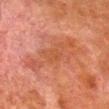workup: no biopsy performed (imaged during a skin exam) | body site: the right lower leg | lighting: cross-polarized | lesion diameter: about 8.5 mm | acquisition: ~15 mm tile from a whole-body skin photo | patient: male, about 80 years old.